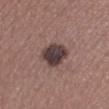From the right lower leg.
The subject is a female aged around 60.
Cropped from a total-body skin-imaging series; the visible field is about 15 mm.
This is a white-light tile.